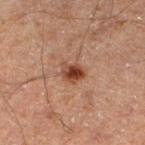  biopsy_status: not biopsied; imaged during a skin examination
  automated_metrics:
    cielab_L: 37
    cielab_a: 22
    cielab_b: 28
    vs_skin_darker_L: 12.0
    vs_skin_contrast_norm: 10.5
    border_irregularity_0_10: 2.5
    color_variation_0_10: 5.5
    peripheral_color_asymmetry: 2.0
  site: left lower leg
  lesion_size:
    long_diameter_mm_approx: 2.5
  patient:
    sex: male
    age_approx: 65
  image:
    source: total-body photography crop
    field_of_view_mm: 15